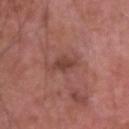Recorded during total-body skin imaging; not selected for excision or biopsy.
From the head or neck.
The total-body-photography lesion software estimated a lesion color around L≈42 a*≈23 b*≈25 in CIELAB, roughly 9 lightness units darker than nearby skin, and a normalized border contrast of about 7. And it measured a border-irregularity rating of about 4/10, internal color variation of about 1.5 on a 0–10 scale, and radial color variation of about 0.5.
Cropped from a whole-body photographic skin survey; the tile spans about 15 mm.
A male patient, approximately 55 years of age.
About 3 mm across.